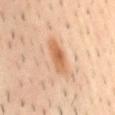Recorded during total-body skin imaging; not selected for excision or biopsy.
A male patient, aged 38–42.
Imaged with cross-polarized lighting.
The lesion is on the mid back.
The total-body-photography lesion software estimated a border-irregularity rating of about 3.5/10, a color-variation rating of about 3.5/10, and peripheral color asymmetry of about 1. And it measured an automated nevus-likeness rating near 95 out of 100 and a detector confidence of about 100 out of 100 that the crop contains a lesion.
A region of skin cropped from a whole-body photographic capture, roughly 15 mm wide.